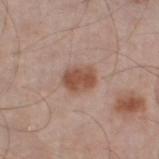notes — imaged on a skin check; not biopsied | site — the left thigh | automated metrics — an eccentricity of roughly 0.6 and a shape-asymmetry score of about 0.15 (0 = symmetric); a color-variation rating of about 3.5/10 and peripheral color asymmetry of about 1 | lesion diameter — ≈3.5 mm | image source — ~15 mm tile from a whole-body skin photo | subject — male, aged around 60.